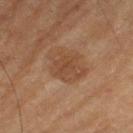<case>
<biopsy_status>not biopsied; imaged during a skin examination</biopsy_status>
<lighting>cross-polarized</lighting>
<patient>
  <sex>male</sex>
  <age_approx>60</age_approx>
</patient>
<site>left thigh</site>
<image>
  <source>total-body photography crop</source>
  <field_of_view_mm>15</field_of_view_mm>
</image>
<automated_metrics>
  <eccentricity>0.55</eccentricity>
  <shape_asymmetry>0.3</shape_asymmetry>
  <border_irregularity_0_10>3.5</border_irregularity_0_10>
  <nevus_likeness_0_100>5</nevus_likeness_0_100>
  <lesion_detection_confidence_0_100>100</lesion_detection_confidence_0_100>
</automated_metrics>
</case>